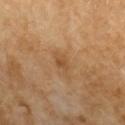Assessment:
Part of a total-body skin-imaging series; this lesion was reviewed on a skin check and was not flagged for biopsy.
Clinical summary:
The lesion is located on the arm. The lesion's longest dimension is about 2.5 mm. Captured under cross-polarized illumination. The patient is a female aged around 70. A 15 mm close-up tile from a total-body photography series done for melanoma screening. The lesion-visualizer software estimated a footprint of about 3.5 mm² and an eccentricity of roughly 0.8. The analysis additionally found a border-irregularity index near 3/10, a color-variation rating of about 1.5/10, and peripheral color asymmetry of about 0.5. It also reported a classifier nevus-likeness of about 0/100 and a detector confidence of about 100 out of 100 that the crop contains a lesion.